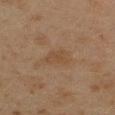Assessment: No biopsy was performed on this lesion — it was imaged during a full skin examination and was not determined to be concerning. Background: Automated image analysis of the tile measured a lesion area of about 4.5 mm², an eccentricity of roughly 0.8, and a symmetry-axis asymmetry near 0.3. And it measured a mean CIELAB color near L≈37 a*≈14 b*≈26 and about 4 CIELAB-L* units darker than the surrounding skin. It also reported a border-irregularity rating of about 3/10, a within-lesion color-variation index near 1.5/10, and radial color variation of about 0.5. A lesion tile, about 15 mm wide, cut from a 3D total-body photograph. Imaged with cross-polarized lighting. A male subject, roughly 45 years of age. On the right upper arm. Approximately 3 mm at its widest.